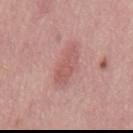Q: Was this lesion biopsied?
A: total-body-photography surveillance lesion; no biopsy
Q: Automated lesion metrics?
A: a lesion color around L≈57 a*≈25 b*≈23 in CIELAB, a lesion–skin lightness drop of about 8, and a normalized lesion–skin contrast near 5.5; border irregularity of about 3 on a 0–10 scale, internal color variation of about 2 on a 0–10 scale, and peripheral color asymmetry of about 0.5
Q: How was this image acquired?
A: ~15 mm crop, total-body skin-cancer survey
Q: Lesion size?
A: ~4.5 mm (longest diameter)
Q: What is the anatomic site?
A: the mid back
Q: Who is the patient?
A: male, in their mid-70s